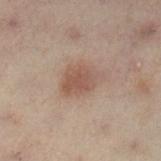Part of a total-body skin-imaging series; this lesion was reviewed on a skin check and was not flagged for biopsy. From the leg. A female patient, about 55 years old. Cropped from a total-body skin-imaging series; the visible field is about 15 mm.